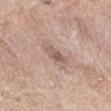Q: Is there a histopathology result?
A: imaged on a skin check; not biopsied
Q: Patient demographics?
A: female, aged 58–62
Q: How was this image acquired?
A: ~15 mm tile from a whole-body skin photo
Q: What is the anatomic site?
A: the left thigh